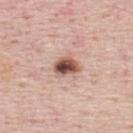{
  "biopsy_status": "not biopsied; imaged during a skin examination",
  "image": {
    "source": "total-body photography crop",
    "field_of_view_mm": 15
  },
  "lesion_size": {
    "long_diameter_mm_approx": 3.5
  },
  "patient": {
    "sex": "male",
    "age_approx": 45
  },
  "automated_metrics": {
    "eccentricity": 0.7,
    "shape_asymmetry": 0.25,
    "border_irregularity_0_10": 2.5,
    "color_variation_0_10": 9.0,
    "nevus_likeness_0_100": 100
  },
  "lighting": "white-light",
  "site": "mid back"
}